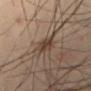Captured during whole-body skin photography for melanoma surveillance; the lesion was not biopsied.
Cropped from a total-body skin-imaging series; the visible field is about 15 mm.
A male subject about 55 years old.
Captured under cross-polarized illumination.
Longest diameter approximately 3 mm.
Located on the leg.
An algorithmic analysis of the crop reported an area of roughly 5 mm², a shape eccentricity near 0.8, and two-axis asymmetry of about 0.25. The analysis additionally found an average lesion color of about L≈41 a*≈15 b*≈25 (CIELAB), a lesion–skin lightness drop of about 10, and a normalized border contrast of about 8.5. The software also gave border irregularity of about 2.5 on a 0–10 scale and a peripheral color-asymmetry measure near 1. The analysis additionally found a nevus-likeness score of about 90/100 and lesion-presence confidence of about 100/100.